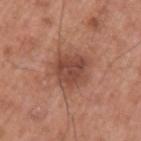Context: The subject is a male aged around 55. The lesion is on the left upper arm. A close-up tile cropped from a whole-body skin photograph, about 15 mm across.